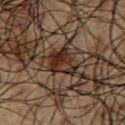Context: Captured under cross-polarized illumination. A male patient about 60 years old. A roughly 15 mm field-of-view crop from a total-body skin photograph. On the chest. Longest diameter approximately 3.5 mm.